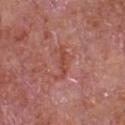* follow-up · catalogued during a skin exam; not biopsied